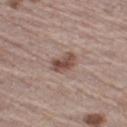| field | value |
|---|---|
| follow-up | catalogued during a skin exam; not biopsied |
| subject | male, roughly 65 years of age |
| tile lighting | white-light illumination |
| automated metrics | an average lesion color of about L≈49 a*≈18 b*≈23 (CIELAB) and a normalized border contrast of about 8.5; a within-lesion color-variation index near 4.5/10; a classifier nevus-likeness of about 85/100 and lesion-presence confidence of about 100/100 |
| body site | the leg |
| acquisition | ~15 mm tile from a whole-body skin photo |